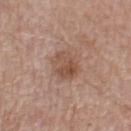{
  "biopsy_status": "not biopsied; imaged during a skin examination",
  "image": {
    "source": "total-body photography crop",
    "field_of_view_mm": 15
  },
  "site": "left upper arm",
  "patient": {
    "sex": "male",
    "age_approx": 65
  }
}